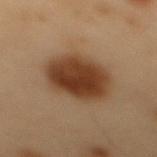Part of a total-body skin-imaging series; this lesion was reviewed on a skin check and was not flagged for biopsy.
A male subject in their mid- to late 50s.
A roughly 15 mm field-of-view crop from a total-body skin photograph.
Located on the mid back.
Imaged with cross-polarized lighting.
Automated image analysis of the tile measured a lesion color around L≈31 a*≈16 b*≈27 in CIELAB. The software also gave border irregularity of about 1.5 on a 0–10 scale and radial color variation of about 1. The software also gave an automated nevus-likeness rating near 100 out of 100 and lesion-presence confidence of about 100/100.